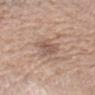{"biopsy_status": "not biopsied; imaged during a skin examination", "patient": {"sex": "male", "age_approx": 45}, "lighting": "white-light", "automated_metrics": {"eccentricity": 0.45, "shape_asymmetry": 0.25, "nevus_likeness_0_100": 0, "lesion_detection_confidence_0_100": 100}, "image": {"source": "total-body photography crop", "field_of_view_mm": 15}, "site": "head or neck", "lesion_size": {"long_diameter_mm_approx": 3.0}}